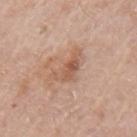Q: Was this lesion biopsied?
A: imaged on a skin check; not biopsied
Q: How large is the lesion?
A: ~4.5 mm (longest diameter)
Q: What kind of image is this?
A: total-body-photography crop, ~15 mm field of view
Q: Illumination type?
A: white-light illumination
Q: Where on the body is the lesion?
A: the left upper arm
Q: Patient demographics?
A: male, roughly 65 years of age
Q: Automated lesion metrics?
A: a border-irregularity index near 6/10 and peripheral color asymmetry of about 1.5